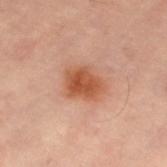Captured during whole-body skin photography for melanoma surveillance; the lesion was not biopsied.
From the right leg.
Captured under cross-polarized illumination.
About 4 mm across.
Automated tile analysis of the lesion measured a footprint of about 10 mm² and an outline eccentricity of about 0.65 (0 = round, 1 = elongated). It also reported a border-irregularity index near 2/10, a color-variation rating of about 3.5/10, and peripheral color asymmetry of about 1. It also reported a classifier nevus-likeness of about 100/100 and a detector confidence of about 100 out of 100 that the crop contains a lesion.
Cropped from a total-body skin-imaging series; the visible field is about 15 mm.
A female subject aged 58–62.